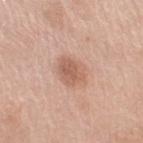{
  "biopsy_status": "not biopsied; imaged during a skin examination"
}